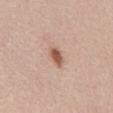notes = imaged on a skin check; not biopsied | location = the front of the torso | size = ~3 mm (longest diameter) | imaging modality = total-body-photography crop, ~15 mm field of view | patient = female, aged 28 to 32.